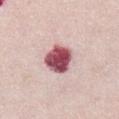The lesion was photographed on a routine skin check and not biopsied; there is no pathology result.
A female patient, aged approximately 65.
Automated image analysis of the tile measured an average lesion color of about L≈52 a*≈31 b*≈17 (CIELAB). It also reported a nevus-likeness score of about 40/100 and lesion-presence confidence of about 100/100.
The lesion is located on the front of the torso.
A lesion tile, about 15 mm wide, cut from a 3D total-body photograph.